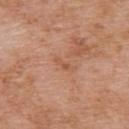Imaged during a routine full-body skin examination; the lesion was not biopsied and no histopathology is available. A roughly 15 mm field-of-view crop from a total-body skin photograph. On the upper back. A male patient, about 75 years old.